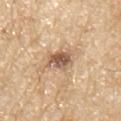Part of a total-body skin-imaging series; this lesion was reviewed on a skin check and was not flagged for biopsy. The lesion is on the arm. Cropped from a whole-body photographic skin survey; the tile spans about 15 mm. This is a white-light tile. A male subject, aged 68 to 72. The lesion-visualizer software estimated an area of roughly 7.5 mm². The analysis additionally found a border-irregularity rating of about 3/10, internal color variation of about 6 on a 0–10 scale, and a peripheral color-asymmetry measure near 2. The software also gave an automated nevus-likeness rating near 85 out of 100.